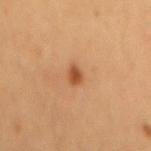No biopsy was performed on this lesion — it was imaged during a full skin examination and was not determined to be concerning.
A male subject, aged approximately 60.
A 15 mm crop from a total-body photograph taken for skin-cancer surveillance.
From the mid back.
This is a cross-polarized tile.